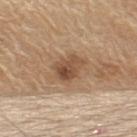biopsy status — catalogued during a skin exam; not biopsied.